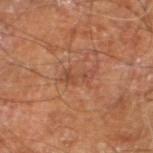The lesion was photographed on a routine skin check and not biopsied; there is no pathology result.
A roughly 15 mm field-of-view crop from a total-body skin photograph.
The subject is a male aged around 60.
The lesion is located on the leg.
About 3.5 mm across.